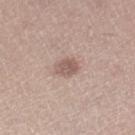Q: Was this lesion biopsied?
A: imaged on a skin check; not biopsied
Q: Patient demographics?
A: female, approximately 45 years of age
Q: What is the imaging modality?
A: 15 mm crop, total-body photography
Q: Lesion location?
A: the right lower leg
Q: How large is the lesion?
A: ~2.5 mm (longest diameter)
Q: Automated lesion metrics?
A: an average lesion color of about L≈57 a*≈17 b*≈24 (CIELAB) and a lesion–skin lightness drop of about 10; a border-irregularity index near 1.5/10 and peripheral color asymmetry of about 1